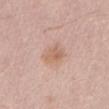Q: Was a biopsy performed?
A: total-body-photography surveillance lesion; no biopsy
Q: What lighting was used for the tile?
A: white-light illumination
Q: What is the anatomic site?
A: the mid back
Q: How large is the lesion?
A: ~3 mm (longest diameter)
Q: What are the patient's age and sex?
A: male, aged approximately 65
Q: Automated lesion metrics?
A: a lesion area of about 5.5 mm² and an outline eccentricity of about 0.75 (0 = round, 1 = elongated); a mean CIELAB color near L≈62 a*≈20 b*≈29 and about 8 CIELAB-L* units darker than the surrounding skin
Q: What kind of image is this?
A: ~15 mm tile from a whole-body skin photo